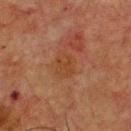The tile uses cross-polarized illumination. A male patient, in their mid-70s. Longest diameter approximately 2.5 mm. Cropped from a total-body skin-imaging series; the visible field is about 15 mm. Automated tile analysis of the lesion measured an average lesion color of about L≈34 a*≈20 b*≈30 (CIELAB) and a lesion–skin lightness drop of about 4. Located on the chest.